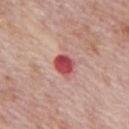An algorithmic analysis of the crop reported a lesion area of about 5 mm² and an eccentricity of roughly 0.6. And it measured a border-irregularity index near 1/10 and a peripheral color-asymmetry measure near 1.
About 2.5 mm across.
Cropped from a whole-body photographic skin survey; the tile spans about 15 mm.
The subject is a male aged around 75.
This is a white-light tile.
From the mid back.